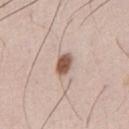notes = total-body-photography surveillance lesion; no biopsy
subject = male, about 35 years old
image = total-body-photography crop, ~15 mm field of view
site = the abdomen